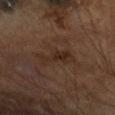Impression: Part of a total-body skin-imaging series; this lesion was reviewed on a skin check and was not flagged for biopsy. Background: Captured under cross-polarized illumination. A male patient, aged around 65. The lesion is on the left forearm. Longest diameter approximately 4 mm. Cropped from a total-body skin-imaging series; the visible field is about 15 mm. Automated tile analysis of the lesion measured roughly 6 lightness units darker than nearby skin and a lesion-to-skin contrast of about 6.5 (normalized; higher = more distinct). It also reported a border-irregularity index near 6/10 and a within-lesion color-variation index near 2.5/10.